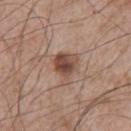Impression:
This lesion was catalogued during total-body skin photography and was not selected for biopsy.
Clinical summary:
This image is a 15 mm lesion crop taken from a total-body photograph. Located on the left upper arm. The subject is a male aged 63–67. Longest diameter approximately 4 mm.